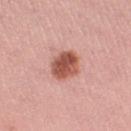Assessment: The lesion was photographed on a routine skin check and not biopsied; there is no pathology result. Background: A female patient in their mid-30s. A 15 mm close-up extracted from a 3D total-body photography capture. From the right thigh.